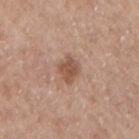biopsy status = total-body-photography surveillance lesion; no biopsy
site = the right upper arm
subject = male, aged around 70
lesion diameter = about 3.5 mm
imaging modality = ~15 mm crop, total-body skin-cancer survey